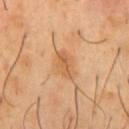workup: imaged on a skin check; not biopsied
patient: male, aged 48–52
anatomic site: the chest
acquisition: ~15 mm crop, total-body skin-cancer survey